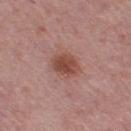{"biopsy_status": "not biopsied; imaged during a skin examination", "patient": {"sex": "female", "age_approx": 50}, "lesion_size": {"long_diameter_mm_approx": 3.5}, "automated_metrics": {"border_irregularity_0_10": 1.5, "color_variation_0_10": 3.5, "peripheral_color_asymmetry": 1.0, "nevus_likeness_0_100": 90, "lesion_detection_confidence_0_100": 100}, "lighting": "white-light", "site": "right thigh", "image": {"source": "total-body photography crop", "field_of_view_mm": 15}}